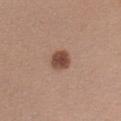{"biopsy_status": "not biopsied; imaged during a skin examination", "lesion_size": {"long_diameter_mm_approx": 3.0}, "site": "arm", "image": {"source": "total-body photography crop", "field_of_view_mm": 15}, "patient": {"sex": "female", "age_approx": 30}}